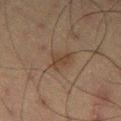Captured during whole-body skin photography for melanoma surveillance; the lesion was not biopsied.
On the left thigh.
A close-up tile cropped from a whole-body skin photograph, about 15 mm across.
The subject is a male about 60 years old.
Automated image analysis of the tile measured a border-irregularity rating of about 3.5/10 and a peripheral color-asymmetry measure near 0.5. The analysis additionally found a nevus-likeness score of about 20/100 and a detector confidence of about 100 out of 100 that the crop contains a lesion.
This is a cross-polarized tile.